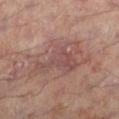Q: Is there a histopathology result?
A: total-body-photography surveillance lesion; no biopsy
Q: Lesion size?
A: about 9 mm
Q: What did automated image analysis measure?
A: radial color variation of about 1.5; an automated nevus-likeness rating near 0 out of 100
Q: What lighting was used for the tile?
A: cross-polarized illumination
Q: What kind of image is this?
A: total-body-photography crop, ~15 mm field of view
Q: Patient demographics?
A: male, roughly 55 years of age
Q: Lesion location?
A: the left lower leg